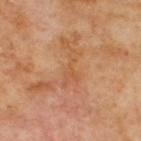| field | value |
|---|---|
| notes | total-body-photography surveillance lesion; no biopsy |
| lesion size | ~4.5 mm (longest diameter) |
| image | ~15 mm tile from a whole-body skin photo |
| tile lighting | cross-polarized |
| anatomic site | the back |
| subject | male, aged approximately 70 |
| TBP lesion metrics | a color-variation rating of about 2/10; an automated nevus-likeness rating near 0 out of 100 and lesion-presence confidence of about 100/100 |